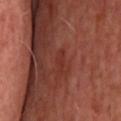Part of a total-body skin-imaging series; this lesion was reviewed on a skin check and was not flagged for biopsy. Longest diameter approximately 2.5 mm. A male subject approximately 65 years of age. From the back. Automated image analysis of the tile measured a lesion area of about 2.5 mm², an eccentricity of roughly 0.9, and a symmetry-axis asymmetry near 0.4. The software also gave an average lesion color of about L≈32 a*≈27 b*≈26 (CIELAB) and about 5 CIELAB-L* units darker than the surrounding skin. The software also gave a detector confidence of about 95 out of 100 that the crop contains a lesion. A lesion tile, about 15 mm wide, cut from a 3D total-body photograph.